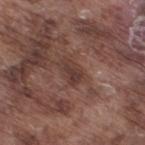Assessment: Recorded during total-body skin imaging; not selected for excision or biopsy. Background: The lesion is on the right thigh. This is a white-light tile. A male patient, approximately 75 years of age. A lesion tile, about 15 mm wide, cut from a 3D total-body photograph. The recorded lesion diameter is about 3 mm.